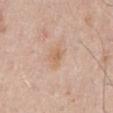Clinical impression: No biopsy was performed on this lesion — it was imaged during a full skin examination and was not determined to be concerning. Context: Located on the mid back. The total-body-photography lesion software estimated roughly 6 lightness units darker than nearby skin and a normalized lesion–skin contrast near 5.5. And it measured a border-irregularity rating of about 2.5/10, internal color variation of about 2.5 on a 0–10 scale, and peripheral color asymmetry of about 1. And it measured lesion-presence confidence of about 100/100. Captured under white-light illumination. The patient is a male aged 38 to 42. Cropped from a total-body skin-imaging series; the visible field is about 15 mm. Approximately 2.5 mm at its widest.